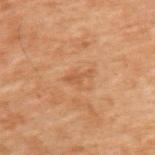This lesion was catalogued during total-body skin photography and was not selected for biopsy. Measured at roughly 3 mm in maximum diameter. This is a cross-polarized tile. The lesion is located on the upper back. A male subject, about 70 years old. A 15 mm crop from a total-body photograph taken for skin-cancer surveillance.